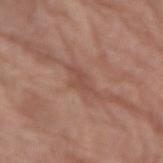Impression: Part of a total-body skin-imaging series; this lesion was reviewed on a skin check and was not flagged for biopsy. Image and clinical context: An algorithmic analysis of the crop reported a footprint of about 4 mm², an outline eccentricity of about 0.9 (0 = round, 1 = elongated), and two-axis asymmetry of about 0.45. The lesion is located on the right forearm. A 15 mm close-up extracted from a 3D total-body photography capture. A female patient aged 78 to 82. About 4 mm across.